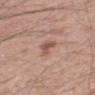Findings:
* follow-up: no biopsy performed (imaged during a skin exam)